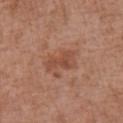Case summary:
• follow-up: imaged on a skin check; not biopsied
• image: 15 mm crop, total-body photography
• patient: female, aged 73–77
• lesion size: ≈4 mm
• body site: the chest
• illumination: white-light illumination